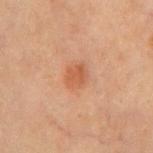Clinical impression:
Imaged during a routine full-body skin examination; the lesion was not biopsied and no histopathology is available.
Acquisition and patient details:
The subject is a male aged 68–72. The recorded lesion diameter is about 3 mm. Automated image analysis of the tile measured a lesion area of about 5 mm², a shape eccentricity near 0.6, and a symmetry-axis asymmetry near 0.25. And it measured a mean CIELAB color near L≈43 a*≈21 b*≈29, a lesion–skin lightness drop of about 7, and a normalized lesion–skin contrast near 6. The analysis additionally found a border-irregularity index near 2.5/10, a color-variation rating of about 1.5/10, and peripheral color asymmetry of about 0.5. The software also gave an automated nevus-likeness rating near 75 out of 100. This image is a 15 mm lesion crop taken from a total-body photograph. On the chest. Captured under cross-polarized illumination.